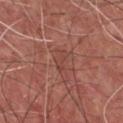<lesion>
  <biopsy_status>not biopsied; imaged during a skin examination</biopsy_status>
  <patient>
    <sex>male</sex>
    <age_approx>75</age_approx>
  </patient>
  <image>
    <source>total-body photography crop</source>
    <field_of_view_mm>15</field_of_view_mm>
  </image>
  <site>chest</site>
</lesion>